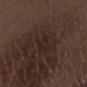follow-up: total-body-photography surveillance lesion; no biopsy
acquisition: ~15 mm tile from a whole-body skin photo
site: the right forearm
subject: male, in their 70s
diameter: about 4 mm
TBP lesion metrics: an outline eccentricity of about 0.9 (0 = round, 1 = elongated) and two-axis asymmetry of about 0.5; a lesion color around L≈22 a*≈15 b*≈16 in CIELAB, about 4 CIELAB-L* units darker than the surrounding skin, and a lesion-to-skin contrast of about 5.5 (normalized; higher = more distinct)